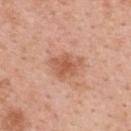Impression:
Captured during whole-body skin photography for melanoma surveillance; the lesion was not biopsied.
Clinical summary:
Cropped from a whole-body photographic skin survey; the tile spans about 15 mm. The subject is a female approximately 50 years of age. From the upper back.